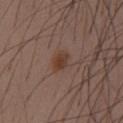Assessment:
Captured during whole-body skin photography for melanoma surveillance; the lesion was not biopsied.
Acquisition and patient details:
A male subject, in their 40s. A lesion tile, about 15 mm wide, cut from a 3D total-body photograph. The lesion is located on the chest.